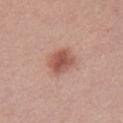Q: Was a biopsy performed?
A: imaged on a skin check; not biopsied
Q: Lesion location?
A: the chest
Q: What is the imaging modality?
A: ~15 mm tile from a whole-body skin photo
Q: Automated lesion metrics?
A: a lesion area of about 8 mm² and a shape eccentricity near 0.7
Q: How large is the lesion?
A: ≈3.5 mm
Q: Illumination type?
A: white-light
Q: Patient demographics?
A: female, approximately 35 years of age